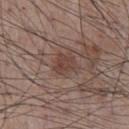Clinical impression: Part of a total-body skin-imaging series; this lesion was reviewed on a skin check and was not flagged for biopsy. Acquisition and patient details: The subject is a male approximately 55 years of age. The lesion is located on the chest. Automated tile analysis of the lesion measured a border-irregularity rating of about 3/10, internal color variation of about 3 on a 0–10 scale, and a peripheral color-asymmetry measure near 1. The software also gave a classifier nevus-likeness of about 25/100. A close-up tile cropped from a whole-body skin photograph, about 15 mm across. Longest diameter approximately 3 mm.